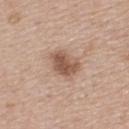site: upper back
patient:
  sex: female
  age_approx: 45
lesion_size:
  long_diameter_mm_approx: 4.0
lighting: white-light
image:
  source: total-body photography crop
  field_of_view_mm: 15